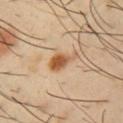  automated_metrics:
    area_mm2_approx: 5.5
    vs_skin_darker_L: 13.0
    border_irregularity_0_10: 4.5
    color_variation_0_10: 3.5
    peripheral_color_asymmetry: 1.0
    nevus_likeness_0_100: 90
    lesion_detection_confidence_0_100: 100
  patient:
    sex: male
    age_approx: 40
  site: left upper arm
  lesion_size:
    long_diameter_mm_approx: 4.0
  image:
    source: total-body photography crop
    field_of_view_mm: 15
  lighting: cross-polarized